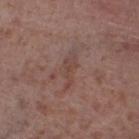Recorded during total-body skin imaging; not selected for excision or biopsy. A lesion tile, about 15 mm wide, cut from a 3D total-body photograph. Located on the left lower leg. A male subject, roughly 55 years of age.